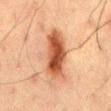follow-up = imaged on a skin check; not biopsied | size = about 6.5 mm | anatomic site = the mid back | TBP lesion metrics = a border-irregularity index near 3/10 | image = total-body-photography crop, ~15 mm field of view | subject = male, aged 58–62.